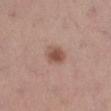follow-up — catalogued during a skin exam; not biopsied | image — ~15 mm tile from a whole-body skin photo | illumination — white-light illumination | patient — female, about 55 years old | image-analysis metrics — an eccentricity of roughly 0.6 and a symmetry-axis asymmetry near 0.25; a border-irregularity index near 2/10, a within-lesion color-variation index near 2.5/10, and peripheral color asymmetry of about 1; a classifier nevus-likeness of about 95/100 and a lesion-detection confidence of about 100/100 | body site — the leg.